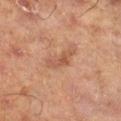Notes:
- biopsy status · total-body-photography surveillance lesion; no biopsy
- diameter · ≈4 mm
- tile lighting · cross-polarized
- body site · the left lower leg
- patient · male, in their mid- to late 60s
- acquisition · total-body-photography crop, ~15 mm field of view